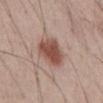biopsy_status: not biopsied; imaged during a skin examination
lesion_size:
  long_diameter_mm_approx: 4.0
site: abdomen
image:
  source: total-body photography crop
  field_of_view_mm: 15
lighting: white-light
patient:
  sex: male
  age_approx: 40